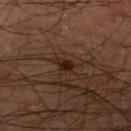Captured during whole-body skin photography for melanoma surveillance; the lesion was not biopsied.
The lesion is on the left thigh.
This image is a 15 mm lesion crop taken from a total-body photograph.
Captured under cross-polarized illumination.
The lesion-visualizer software estimated border irregularity of about 3 on a 0–10 scale and radial color variation of about 0.5. The analysis additionally found a classifier nevus-likeness of about 60/100 and a lesion-detection confidence of about 100/100.
A male subject aged approximately 60.